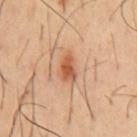Assessment:
Part of a total-body skin-imaging series; this lesion was reviewed on a skin check and was not flagged for biopsy.
Image and clinical context:
About 3 mm across. On the front of the torso. The lesion-visualizer software estimated an area of roughly 4.5 mm², an outline eccentricity of about 0.8 (0 = round, 1 = elongated), and a shape-asymmetry score of about 0.25 (0 = symmetric). The software also gave an automated nevus-likeness rating near 95 out of 100 and lesion-presence confidence of about 100/100. Captured under cross-polarized illumination. This image is a 15 mm lesion crop taken from a total-body photograph. A male subject, roughly 55 years of age.